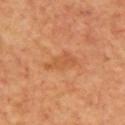Imaged during a routine full-body skin examination; the lesion was not biopsied and no histopathology is available.
The recorded lesion diameter is about 4.5 mm.
An algorithmic analysis of the crop reported a lesion area of about 5.5 mm², an eccentricity of roughly 0.9, and two-axis asymmetry of about 0.4. It also reported an automated nevus-likeness rating near 0 out of 100 and a lesion-detection confidence of about 100/100.
A male subject aged around 70.
The lesion is on the upper back.
A lesion tile, about 15 mm wide, cut from a 3D total-body photograph.
The tile uses cross-polarized illumination.